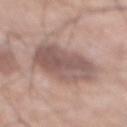Part of a total-body skin-imaging series; this lesion was reviewed on a skin check and was not flagged for biopsy. This is a white-light tile. On the abdomen. A male patient, aged approximately 70. A region of skin cropped from a whole-body photographic capture, roughly 15 mm wide. Automated tile analysis of the lesion measured an average lesion color of about L≈54 a*≈17 b*≈21 (CIELAB) and a lesion–skin lightness drop of about 11. The analysis additionally found a border-irregularity rating of about 1.5/10 and a color-variation rating of about 4.5/10.